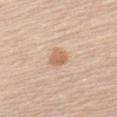{
  "biopsy_status": "not biopsied; imaged during a skin examination",
  "patient": {
    "sex": "female",
    "age_approx": 50
  },
  "image": {
    "source": "total-body photography crop",
    "field_of_view_mm": 15
  },
  "site": "left upper arm"
}